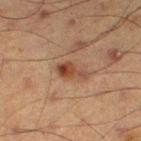Clinical impression: No biopsy was performed on this lesion — it was imaged during a full skin examination and was not determined to be concerning. Background: A 15 mm close-up tile from a total-body photography series done for melanoma screening. A male subject roughly 60 years of age. From the leg.